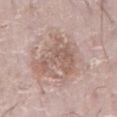• size — ~5.5 mm (longest diameter)
• image — total-body-photography crop, ~15 mm field of view
• patient — male, about 75 years old
• body site — the abdomen
• lighting — white-light